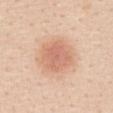Notes:
• biopsy status — total-body-photography surveillance lesion; no biopsy
• TBP lesion metrics — about 10 CIELAB-L* units darker than the surrounding skin and a normalized border contrast of about 6
• acquisition — 15 mm crop, total-body photography
• patient — female, aged 43–47
• anatomic site — the front of the torso
• size — ~4.5 mm (longest diameter)